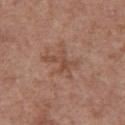Automated image analysis of the tile measured a shape eccentricity near 0.7 and a shape-asymmetry score of about 0.5 (0 = symmetric). And it measured an average lesion color of about L≈49 a*≈20 b*≈28 (CIELAB) and a normalized border contrast of about 5.5. The software also gave a border-irregularity rating of about 8.5/10 and radial color variation of about 1.
A 15 mm close-up extracted from a 3D total-body photography capture.
The recorded lesion diameter is about 4.5 mm.
A female subject in their mid-70s.
This is a white-light tile.
The lesion is on the front of the torso.